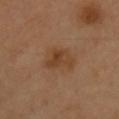Findings:
* lighting · cross-polarized illumination
* subject · male, aged 38–42
* lesion diameter · ≈4 mm
* body site · the chest
* imaging modality · 15 mm crop, total-body photography